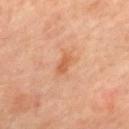Clinical impression:
Captured during whole-body skin photography for melanoma surveillance; the lesion was not biopsied.
Image and clinical context:
The patient is a male aged 63 to 67. Longest diameter approximately 2.5 mm. A 15 mm crop from a total-body photograph taken for skin-cancer surveillance. Located on the mid back. Captured under cross-polarized illumination.